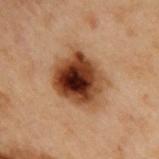Context: From the upper back. Cropped from a whole-body photographic skin survey; the tile spans about 15 mm. The patient is a male about 55 years old.